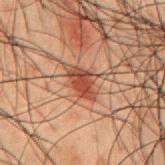A 15 mm crop from a total-body photograph taken for skin-cancer surveillance.
A male subject aged around 50.
This is a cross-polarized tile.
Automated image analysis of the tile measured an average lesion color of about L≈35 a*≈23 b*≈26 (CIELAB) and roughly 11 lightness units darker than nearby skin. The software also gave border irregularity of about 3 on a 0–10 scale and radial color variation of about 1.5. The software also gave a classifier nevus-likeness of about 95/100 and lesion-presence confidence of about 100/100.
Measured at roughly 3.5 mm in maximum diameter.
Located on the mid back.